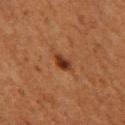follow-up = total-body-photography surveillance lesion; no biopsy.